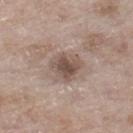Assessment: The lesion was photographed on a routine skin check and not biopsied; there is no pathology result. Context: About 3.5 mm across. A 15 mm crop from a total-body photograph taken for skin-cancer surveillance. Located on the right lower leg. Automated image analysis of the tile measured an area of roughly 9.5 mm², a shape eccentricity near 0.35, and a symmetry-axis asymmetry near 0.25. It also reported an average lesion color of about L≈51 a*≈14 b*≈23 (CIELAB), about 11 CIELAB-L* units darker than the surrounding skin, and a lesion-to-skin contrast of about 8 (normalized; higher = more distinct). The software also gave a classifier nevus-likeness of about 25/100 and lesion-presence confidence of about 100/100. The patient is a female aged approximately 75.